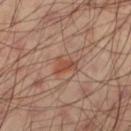Q: Is there a histopathology result?
A: no biopsy performed (imaged during a skin exam)
Q: What did automated image analysis measure?
A: an area of roughly 4 mm², a shape eccentricity near 0.75, and a symmetry-axis asymmetry near 0.45; border irregularity of about 4.5 on a 0–10 scale, a within-lesion color-variation index near 1.5/10, and a peripheral color-asymmetry measure near 0.5
Q: What lighting was used for the tile?
A: cross-polarized
Q: Patient demographics?
A: male, aged 58–62
Q: What is the anatomic site?
A: the left thigh
Q: What kind of image is this?
A: total-body-photography crop, ~15 mm field of view
Q: Lesion size?
A: about 2.5 mm